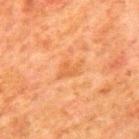Background: The recorded lesion diameter is about 3.5 mm. A lesion tile, about 15 mm wide, cut from a 3D total-body photograph. On the mid back. The patient is a male aged approximately 80. Captured under cross-polarized illumination. The lesion-visualizer software estimated a border-irregularity rating of about 4/10, internal color variation of about 1 on a 0–10 scale, and peripheral color asymmetry of about 0.5.